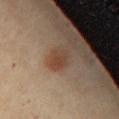Impression: Recorded during total-body skin imaging; not selected for excision or biopsy. Background: From the chest. A female subject, about 45 years old. A 15 mm close-up tile from a total-body photography series done for melanoma screening.